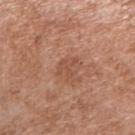The lesion was tiled from a total-body skin photograph and was not biopsied. The subject is a male in their mid- to late 50s. The tile uses white-light illumination. About 2.5 mm across. A roughly 15 mm field-of-view crop from a total-body skin photograph. Automated image analysis of the tile measured a lesion area of about 3.5 mm², an eccentricity of roughly 0.65, and two-axis asymmetry of about 0.6. And it measured a lesion color around L≈51 a*≈23 b*≈31 in CIELAB, a lesion–skin lightness drop of about 7, and a normalized border contrast of about 5. Located on the right upper arm.